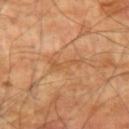Clinical impression: Captured during whole-body skin photography for melanoma surveillance; the lesion was not biopsied. Acquisition and patient details: A male subject, aged 68 to 72. A lesion tile, about 15 mm wide, cut from a 3D total-body photograph. The lesion is on the arm. Captured under cross-polarized illumination.